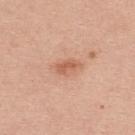{"biopsy_status": "not biopsied; imaged during a skin examination", "lighting": "white-light", "site": "back", "image": {"source": "total-body photography crop", "field_of_view_mm": 15}, "patient": {"sex": "female", "age_approx": 40}}